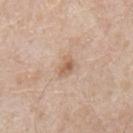notes: catalogued during a skin exam; not biopsied | patient: male, in their mid- to late 60s | site: the mid back | image-analysis metrics: a symmetry-axis asymmetry near 0.2; a nevus-likeness score of about 25/100 | image source: total-body-photography crop, ~15 mm field of view | illumination: white-light.